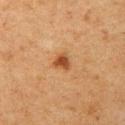Imaged during a routine full-body skin examination; the lesion was not biopsied and no histopathology is available. This is a cross-polarized tile. A 15 mm close-up tile from a total-body photography series done for melanoma screening. From the left upper arm. The recorded lesion diameter is about 2.5 mm. A female subject aged approximately 40.